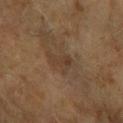Clinical summary: The tile uses cross-polarized illumination. Automated tile analysis of the lesion measured a lesion–skin lightness drop of about 5 and a normalized border contrast of about 5.5. Measured at roughly 3 mm in maximum diameter. This image is a 15 mm lesion crop taken from a total-body photograph. A female patient approximately 60 years of age. The lesion is located on the arm.